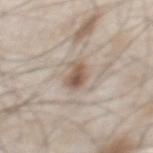follow-up: total-body-photography surveillance lesion; no biopsy
body site: the chest
TBP lesion metrics: a footprint of about 4.5 mm², an outline eccentricity of about 0.8 (0 = round, 1 = elongated), and two-axis asymmetry of about 0.25; an automated nevus-likeness rating near 95 out of 100 and a lesion-detection confidence of about 100/100
patient: male, roughly 55 years of age
lesion diameter: about 3 mm
lighting: white-light
image source: ~15 mm tile from a whole-body skin photo